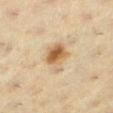biopsy status = no biopsy performed (imaged during a skin exam); site = the left lower leg; image source = ~15 mm tile from a whole-body skin photo; subject = female, aged 38–42; lighting = cross-polarized.